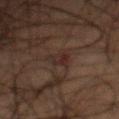<tbp_lesion>
  <biopsy_status>not biopsied; imaged during a skin examination</biopsy_status>
  <automated_metrics>
    <area_mm2_approx>3.5</area_mm2_approx>
    <eccentricity>0.8</eccentricity>
    <shape_asymmetry>0.25</shape_asymmetry>
    <vs_skin_darker_L>5.0</vs_skin_darker_L>
    <vs_skin_contrast_norm>6.5</vs_skin_contrast_norm>
    <border_irregularity_0_10>2.0</border_irregularity_0_10>
    <color_variation_0_10>4.0</color_variation_0_10>
  </automated_metrics>
  <image>
    <source>total-body photography crop</source>
    <field_of_view_mm>15</field_of_view_mm>
  </image>
  <patient>
    <sex>male</sex>
    <age_approx>50</age_approx>
  </patient>
  <site>back</site>
  <lighting>cross-polarized</lighting>
  <lesion_size>
    <long_diameter_mm_approx>2.5</long_diameter_mm_approx>
  </lesion_size>
</tbp_lesion>